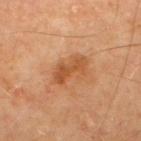  biopsy_status: not biopsied; imaged during a skin examination
  automated_metrics:
    area_mm2_approx: 8.5
    eccentricity: 0.85
    shape_asymmetry: 0.3
    cielab_L: 52
    cielab_a: 25
    cielab_b: 39
    vs_skin_darker_L: 9.0
    color_variation_0_10: 4.0
    peripheral_color_asymmetry: 1.5
  patient:
    sex: male
    age_approx: 65
  site: upper back
  lesion_size:
    long_diameter_mm_approx: 4.5
  image:
    source: total-body photography crop
    field_of_view_mm: 15
  lighting: cross-polarized